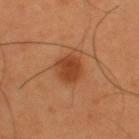No biopsy was performed on this lesion — it was imaged during a full skin examination and was not determined to be concerning. Automated tile analysis of the lesion measured a within-lesion color-variation index near 2.5/10 and radial color variation of about 1. The tile uses cross-polarized illumination. Longest diameter approximately 3.5 mm. A lesion tile, about 15 mm wide, cut from a 3D total-body photograph. A male patient, aged 53–57. From the upper back.